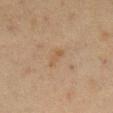<lesion>
<biopsy_status>not biopsied; imaged during a skin examination</biopsy_status>
<patient>
  <sex>female</sex>
  <age_approx>50</age_approx>
</patient>
<lighting>cross-polarized</lighting>
<lesion_size>
  <long_diameter_mm_approx>3.0</long_diameter_mm_approx>
</lesion_size>
<site>leg</site>
<automated_metrics>
  <nevus_likeness_0_100>0</nevus_likeness_0_100>
  <lesion_detection_confidence_0_100>100</lesion_detection_confidence_0_100>
</automated_metrics>
<image>
  <source>total-body photography crop</source>
  <field_of_view_mm>15</field_of_view_mm>
</image>
</lesion>